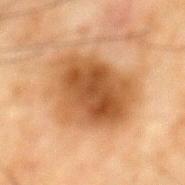Impression: This lesion was catalogued during total-body skin photography and was not selected for biopsy. Acquisition and patient details: The patient is a male about 65 years old. Approximately 7.5 mm at its widest. The lesion is located on the mid back. Cropped from a whole-body photographic skin survey; the tile spans about 15 mm. Captured under cross-polarized illumination.